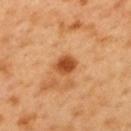Captured during whole-body skin photography for melanoma surveillance; the lesion was not biopsied.
On the mid back.
A 15 mm close-up tile from a total-body photography series done for melanoma screening.
The total-body-photography lesion software estimated an eccentricity of roughly 0.65 and a shape-asymmetry score of about 0.2 (0 = symmetric).
The patient is a male roughly 50 years of age.
The tile uses cross-polarized illumination.
The lesion's longest dimension is about 3 mm.